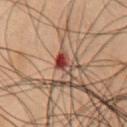Imaged during a routine full-body skin examination; the lesion was not biopsied and no histopathology is available.
A male patient in their mid-50s.
From the chest.
A 15 mm close-up extracted from a 3D total-body photography capture.